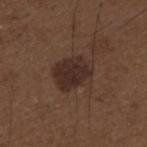notes: total-body-photography surveillance lesion; no biopsy | subject: male, aged 48–52 | TBP lesion metrics: a lesion–skin lightness drop of about 8 and a normalized border contrast of about 9 | site: the upper back | lesion diameter: ~5 mm (longest diameter) | acquisition: ~15 mm tile from a whole-body skin photo | illumination: white-light.